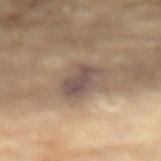This lesion was catalogued during total-body skin photography and was not selected for biopsy. From the left arm. A female subject, aged approximately 80. About 4 mm across. Automated tile analysis of the lesion measured a color-variation rating of about 2.5/10 and radial color variation of about 1. This image is a 15 mm lesion crop taken from a total-body photograph. Captured under cross-polarized illumination.